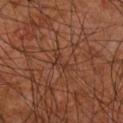No biopsy was performed on this lesion — it was imaged during a full skin examination and was not determined to be concerning.
The lesion is on the right forearm.
A male subject, in their 60s.
Longest diameter approximately 3 mm.
Automated tile analysis of the lesion measured a nevus-likeness score of about 0/100 and a lesion-detection confidence of about 50/100.
A region of skin cropped from a whole-body photographic capture, roughly 15 mm wide.
Imaged with cross-polarized lighting.